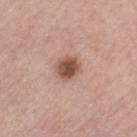biopsy status = imaged on a skin check; not biopsied
body site = the left thigh
diameter = about 3 mm
imaging modality = ~15 mm crop, total-body skin-cancer survey
subject = male, aged around 75